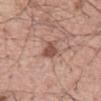A 15 mm crop from a total-body photograph taken for skin-cancer surveillance. The recorded lesion diameter is about 3 mm. The lesion is on the mid back. The tile uses white-light illumination. A male patient, in their mid- to late 50s. An algorithmic analysis of the crop reported an area of roughly 4 mm² and an outline eccentricity of about 0.75 (0 = round, 1 = elongated). And it measured border irregularity of about 3 on a 0–10 scale, a color-variation rating of about 1.5/10, and radial color variation of about 0.5.